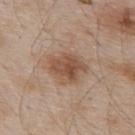Assessment: The lesion was photographed on a routine skin check and not biopsied; there is no pathology result. Background: Captured under white-light illumination. Approximately 4.5 mm at its widest. A male patient, aged around 55. On the upper back. Cropped from a total-body skin-imaging series; the visible field is about 15 mm. The lesion-visualizer software estimated a mean CIELAB color near L≈51 a*≈19 b*≈29.